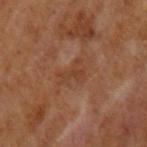biopsy_status: not biopsied; imaged during a skin examination